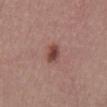biopsy_status: not biopsied; imaged during a skin examination
site: abdomen
lighting: white-light
lesion_size:
  long_diameter_mm_approx: 2.5
image:
  source: total-body photography crop
  field_of_view_mm: 15
patient:
  sex: male
  age_approx: 40